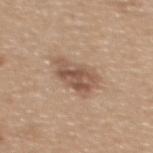This lesion was catalogued during total-body skin photography and was not selected for biopsy. The lesion is on the back. This image is a 15 mm lesion crop taken from a total-body photograph. Automated tile analysis of the lesion measured a lesion color around L≈54 a*≈18 b*≈28 in CIELAB, about 11 CIELAB-L* units darker than the surrounding skin, and a normalized border contrast of about 7.5. Approximately 4.5 mm at its widest. A male patient in their 40s. Captured under white-light illumination.